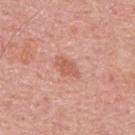subject — male, aged around 65; image — total-body-photography crop, ~15 mm field of view; body site — the upper back.